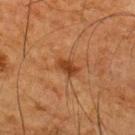Impression:
Imaged during a routine full-body skin examination; the lesion was not biopsied and no histopathology is available.
Context:
Imaged with cross-polarized lighting. About 2.5 mm across. Located on the upper back. A lesion tile, about 15 mm wide, cut from a 3D total-body photograph. A male subject roughly 65 years of age.